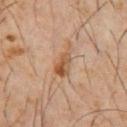No biopsy was performed on this lesion — it was imaged during a full skin examination and was not determined to be concerning.
The tile uses cross-polarized illumination.
The total-body-photography lesion software estimated border irregularity of about 5 on a 0–10 scale, a color-variation rating of about 7/10, and radial color variation of about 2.5. The software also gave an automated nevus-likeness rating near 10 out of 100 and lesion-presence confidence of about 100/100.
The recorded lesion diameter is about 4.5 mm.
A male subject approximately 60 years of age.
This image is a 15 mm lesion crop taken from a total-body photograph.
Located on the chest.